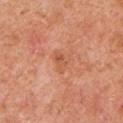biopsy status = no biopsy performed (imaged during a skin exam).